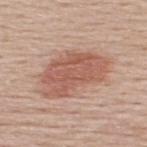The lesion was photographed on a routine skin check and not biopsied; there is no pathology result. The lesion-visualizer software estimated an area of roughly 22 mm², a shape eccentricity near 0.85, and a symmetry-axis asymmetry near 0.3. The software also gave a color-variation rating of about 3.5/10. The subject is a male approximately 60 years of age. The lesion is on the upper back. Measured at roughly 7.5 mm in maximum diameter. A 15 mm crop from a total-body photograph taken for skin-cancer surveillance.